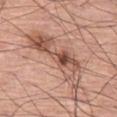anatomic site: the leg; image: ~15 mm crop, total-body skin-cancer survey; subject: male, approximately 75 years of age; illumination: white-light.